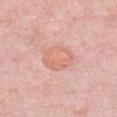<lesion>
<automated_metrics>
  <cielab_L>67</cielab_L>
  <cielab_a>25</cielab_a>
  <cielab_b>29</cielab_b>
  <vs_skin_darker_L>6.0</vs_skin_darker_L>
  <vs_skin_contrast_norm>5.0</vs_skin_contrast_norm>
</automated_metrics>
<patient>
  <sex>female</sex>
  <age_approx>65</age_approx>
</patient>
<image>
  <source>total-body photography crop</source>
  <field_of_view_mm>15</field_of_view_mm>
</image>
<site>chest</site>
</lesion>